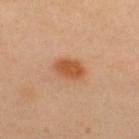Q: How large is the lesion?
A: ≈3.5 mm
Q: How was this image acquired?
A: ~15 mm crop, total-body skin-cancer survey
Q: What lighting was used for the tile?
A: cross-polarized
Q: Where on the body is the lesion?
A: the back
Q: What did automated image analysis measure?
A: an automated nevus-likeness rating near 100 out of 100 and lesion-presence confidence of about 100/100
Q: Who is the patient?
A: male, aged 43 to 47